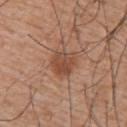workup — imaged on a skin check; not biopsied
image — total-body-photography crop, ~15 mm field of view
lighting — white-light
site — the upper back
lesion diameter — about 3.5 mm
patient — male, approximately 55 years of age